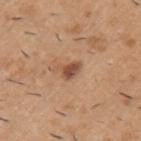The lesion was tiled from a total-body skin photograph and was not biopsied.
Cropped from a total-body skin-imaging series; the visible field is about 15 mm.
On the left upper arm.
A male subject, approximately 40 years of age.